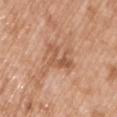lesion diameter = ~5.5 mm (longest diameter) | subject = male, aged 48–52 | body site = the left upper arm | image = ~15 mm crop, total-body skin-cancer survey | illumination = white-light illumination.